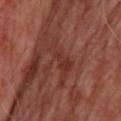follow-up = imaged on a skin check; not biopsied.